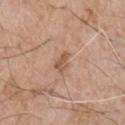No biopsy was performed on this lesion — it was imaged during a full skin examination and was not determined to be concerning. The lesion is located on the right upper arm. A male patient in their mid- to late 70s. Automated tile analysis of the lesion measured an area of roughly 3 mm², an eccentricity of roughly 0.8, and two-axis asymmetry of about 0.4. The analysis additionally found a lesion color around L≈55 a*≈20 b*≈31 in CIELAB, roughly 9 lightness units darker than nearby skin, and a lesion-to-skin contrast of about 6.5 (normalized; higher = more distinct). A roughly 15 mm field-of-view crop from a total-body skin photograph. Imaged with white-light lighting. Approximately 2.5 mm at its widest.